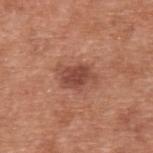notes = catalogued during a skin exam; not biopsied
tile lighting = white-light
imaging modality = ~15 mm crop, total-body skin-cancer survey
automated lesion analysis = a symmetry-axis asymmetry near 0.35; an average lesion color of about L≈47 a*≈25 b*≈29 (CIELAB), about 11 CIELAB-L* units darker than the surrounding skin, and a normalized border contrast of about 8; border irregularity of about 4 on a 0–10 scale, a color-variation rating of about 2.5/10, and radial color variation of about 1; a classifier nevus-likeness of about 85/100
subject = male, in their mid- to late 50s
anatomic site = the upper back
lesion size = about 4 mm